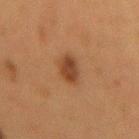  biopsy_status: not biopsied; imaged during a skin examination
  patient:
    sex: female
    age_approx: 50
  lesion_size:
    long_diameter_mm_approx: 3.5
  site: mid back
  automated_metrics:
    cielab_L: 35
    cielab_a: 19
    cielab_b: 29
    vs_skin_darker_L: 9.0
    border_irregularity_0_10: 2.0
    color_variation_0_10: 2.5
    peripheral_color_asymmetry: 1.0
  image:
    source: total-body photography crop
    field_of_view_mm: 15